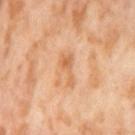subject: female, aged approximately 55 | automated metrics: a footprint of about 4.5 mm², an outline eccentricity of about 0.9 (0 = round, 1 = elongated), and a symmetry-axis asymmetry near 0.6; an average lesion color of about L≈66 a*≈24 b*≈40 (CIELAB) and a lesion-to-skin contrast of about 6 (normalized; higher = more distinct); a border-irregularity rating of about 6.5/10, a within-lesion color-variation index near 2.5/10, and radial color variation of about 1; a classifier nevus-likeness of about 0/100 and a lesion-detection confidence of about 100/100 | lesion diameter: ~4 mm (longest diameter) | location: the left thigh | image source: total-body-photography crop, ~15 mm field of view.